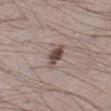biopsy status: total-body-photography surveillance lesion; no biopsy | acquisition: 15 mm crop, total-body photography | lesion diameter: ≈3.5 mm | automated lesion analysis: a border-irregularity rating of about 3.5/10 and a color-variation rating of about 4.5/10 | location: the left lower leg | subject: male, aged 23 to 27 | tile lighting: white-light.